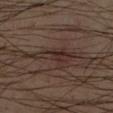Q: Was this lesion biopsied?
A: total-body-photography surveillance lesion; no biopsy
Q: What kind of image is this?
A: ~15 mm crop, total-body skin-cancer survey
Q: What is the anatomic site?
A: the right forearm
Q: How large is the lesion?
A: ≈6 mm
Q: Patient demographics?
A: male, aged 43–47
Q: What lighting was used for the tile?
A: cross-polarized